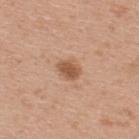{"biopsy_status": "not biopsied; imaged during a skin examination", "patient": {"sex": "male", "age_approx": 30}, "image": {"source": "total-body photography crop", "field_of_view_mm": 15}, "site": "upper back", "lesion_size": {"long_diameter_mm_approx": 2.5}, "lighting": "white-light"}